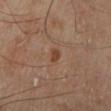Q: Was a biopsy performed?
A: imaged on a skin check; not biopsied
Q: Where on the body is the lesion?
A: the left lower leg
Q: What kind of image is this?
A: 15 mm crop, total-body photography
Q: What did automated image analysis measure?
A: a lesion color around L≈43 a*≈20 b*≈30 in CIELAB, a lesion–skin lightness drop of about 8, and a normalized lesion–skin contrast near 7; border irregularity of about 3 on a 0–10 scale and peripheral color asymmetry of about 0; an automated nevus-likeness rating near 15 out of 100 and a detector confidence of about 100 out of 100 that the crop contains a lesion
Q: Lesion size?
A: ≈2.5 mm
Q: Patient demographics?
A: male, aged around 65
Q: How was the tile lit?
A: cross-polarized illumination